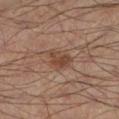No biopsy was performed on this lesion — it was imaged during a full skin examination and was not determined to be concerning.